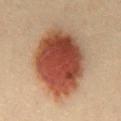Clinical impression: This lesion was catalogued during total-body skin photography and was not selected for biopsy. Clinical summary: Measured at roughly 10.5 mm in maximum diameter. A close-up tile cropped from a whole-body skin photograph, about 15 mm across. Automated image analysis of the tile measured an area of roughly 46 mm² and an outline eccentricity of about 0.8 (0 = round, 1 = elongated). The software also gave an average lesion color of about L≈43 a*≈23 b*≈29 (CIELAB), about 17 CIELAB-L* units darker than the surrounding skin, and a normalized lesion–skin contrast near 13. The software also gave a border-irregularity rating of about 1.5/10 and internal color variation of about 9 on a 0–10 scale. A male patient aged 38–42. From the chest. The tile uses cross-polarized illumination.